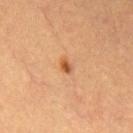* notes: imaged on a skin check; not biopsied
* lighting: cross-polarized illumination
* location: the mid back
* subject: male, aged approximately 65
* automated metrics: about 11 CIELAB-L* units darker than the surrounding skin; a border-irregularity index near 3.5/10, a color-variation rating of about 2.5/10, and a peripheral color-asymmetry measure near 1; a classifier nevus-likeness of about 90/100
* acquisition: total-body-photography crop, ~15 mm field of view
* size: ≈2 mm